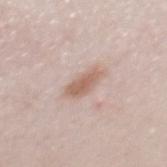No biopsy was performed on this lesion — it was imaged during a full skin examination and was not determined to be concerning. A lesion tile, about 15 mm wide, cut from a 3D total-body photograph. The lesion is on the mid back. A male subject in their mid- to late 20s.